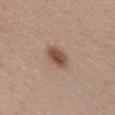Captured during whole-body skin photography for melanoma surveillance; the lesion was not biopsied. This image is a 15 mm lesion crop taken from a total-body photograph. The lesion is located on the head or neck. A female patient aged around 30. Imaged with white-light lighting. The lesion-visualizer software estimated an average lesion color of about L≈50 a*≈19 b*≈28 (CIELAB), a lesion–skin lightness drop of about 13, and a normalized lesion–skin contrast near 9. The software also gave internal color variation of about 4.5 on a 0–10 scale and a peripheral color-asymmetry measure near 1.5. The software also gave an automated nevus-likeness rating near 95 out of 100 and a detector confidence of about 100 out of 100 that the crop contains a lesion. The recorded lesion diameter is about 3.5 mm.